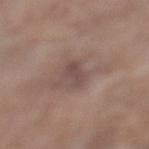The lesion was photographed on a routine skin check and not biopsied; there is no pathology result.
The lesion is on the leg.
Captured under white-light illumination.
The patient is a female aged 73 to 77.
This image is a 15 mm lesion crop taken from a total-body photograph.